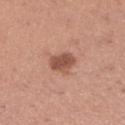Clinical impression:
Captured during whole-body skin photography for melanoma surveillance; the lesion was not biopsied.
Background:
Automated tile analysis of the lesion measured an area of roughly 6 mm² and a symmetry-axis asymmetry near 0.2. The analysis additionally found a border-irregularity rating of about 2/10, internal color variation of about 2 on a 0–10 scale, and peripheral color asymmetry of about 0.5. The lesion is on the right lower leg. This is a white-light tile. About 3 mm across. A female patient, aged approximately 30. A roughly 15 mm field-of-view crop from a total-body skin photograph.